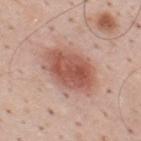Clinical impression: Recorded during total-body skin imaging; not selected for excision or biopsy. Clinical summary: Located on the upper back. A roughly 15 mm field-of-view crop from a total-body skin photograph. Captured under white-light illumination. About 6 mm across. A male patient in their mid- to late 30s.